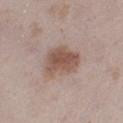Part of a total-body skin-imaging series; this lesion was reviewed on a skin check and was not flagged for biopsy. Cropped from a whole-body photographic skin survey; the tile spans about 15 mm. Located on the left thigh. Automated tile analysis of the lesion measured a mean CIELAB color near L≈53 a*≈18 b*≈25, a lesion–skin lightness drop of about 11, and a lesion-to-skin contrast of about 8 (normalized; higher = more distinct). It also reported a classifier nevus-likeness of about 80/100. Imaged with white-light lighting. The subject is a female aged around 25. About 4.5 mm across.